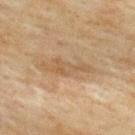<lesion>
<biopsy_status>not biopsied; imaged during a skin examination</biopsy_status>
<automated_metrics>
  <area_mm2_approx>7.0</area_mm2_approx>
  <eccentricity>0.95</eccentricity>
  <shape_asymmetry>0.4</shape_asymmetry>
</automated_metrics>
<site>back</site>
<lighting>cross-polarized</lighting>
<lesion_size>
  <long_diameter_mm_approx>5.5</long_diameter_mm_approx>
</lesion_size>
<patient>
  <sex>female</sex>
  <age_approx>60</age_approx>
</patient>
<image>
  <source>total-body photography crop</source>
  <field_of_view_mm>15</field_of_view_mm>
</image>
</lesion>